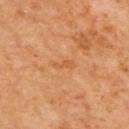Findings:
- follow-up: total-body-photography surveillance lesion; no biopsy
- location: the upper back
- subject: male, aged 63 to 67
- automated lesion analysis: border irregularity of about 4.5 on a 0–10 scale and internal color variation of about 0 on a 0–10 scale; an automated nevus-likeness rating near 0 out of 100 and a lesion-detection confidence of about 100/100
- tile lighting: cross-polarized illumination
- lesion diameter: about 2.5 mm
- acquisition: ~15 mm crop, total-body skin-cancer survey